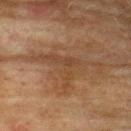notes=imaged on a skin check; not biopsied
imaging modality=~15 mm crop, total-body skin-cancer survey
automated metrics=an area of roughly 8 mm²; a mean CIELAB color near L≈36 a*≈16 b*≈28 and a normalized border contrast of about 5.5
site=the back
subject=male, approximately 75 years of age